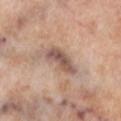Case summary:
• follow-up · no biopsy performed (imaged during a skin exam)
• image · 15 mm crop, total-body photography
• size · ≈4.5 mm
• patient · female, aged approximately 55
• location · the left lower leg
• automated metrics · a lesion color around L≈54 a*≈17 b*≈24 in CIELAB, about 12 CIELAB-L* units darker than the surrounding skin, and a lesion-to-skin contrast of about 8.5 (normalized; higher = more distinct); an automated nevus-likeness rating near 0 out of 100
• tile lighting · cross-polarized illumination